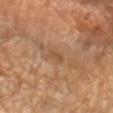Assessment:
Part of a total-body skin-imaging series; this lesion was reviewed on a skin check and was not flagged for biopsy.
Clinical summary:
This image is a 15 mm lesion crop taken from a total-body photograph. The subject is a female roughly 70 years of age. The tile uses cross-polarized illumination. The lesion is located on the left forearm. Longest diameter approximately 2.5 mm.